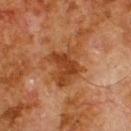lighting: cross-polarized illumination
automated metrics: a mean CIELAB color near L≈34 a*≈21 b*≈31, roughly 8 lightness units darker than nearby skin, and a lesion-to-skin contrast of about 8 (normalized; higher = more distinct); a border-irregularity rating of about 5/10, internal color variation of about 3.5 on a 0–10 scale, and peripheral color asymmetry of about 1.5; a nevus-likeness score of about 0/100 and a lesion-detection confidence of about 100/100
image source: ~15 mm crop, total-body skin-cancer survey
lesion diameter: ≈5.5 mm
subject: male, about 80 years old
location: the back